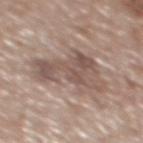biopsy status: catalogued during a skin exam; not biopsied | site: the mid back | acquisition: total-body-photography crop, ~15 mm field of view | subject: male, roughly 65 years of age | automated metrics: a nevus-likeness score of about 10/100 and lesion-presence confidence of about 75/100.